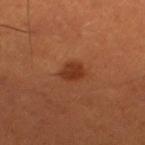<lesion>
  <lighting>cross-polarized</lighting>
  <patient>
    <sex>male</sex>
    <age_approx>50</age_approx>
  </patient>
  <site>right thigh</site>
  <lesion_size>
    <long_diameter_mm_approx>3.0</long_diameter_mm_approx>
  </lesion_size>
  <image>
    <source>total-body photography crop</source>
    <field_of_view_mm>15</field_of_view_mm>
  </image>
</lesion>